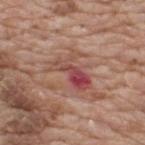Findings:
– follow-up: imaged on a skin check; not biopsied
– image-analysis metrics: a lesion area of about 9.5 mm², a shape eccentricity near 0.8, and two-axis asymmetry of about 0.45; a mean CIELAB color near L≈48 a*≈27 b*≈24 and roughly 10 lightness units darker than nearby skin; internal color variation of about 9.5 on a 0–10 scale and radial color variation of about 3.5
– anatomic site: the back
– subject: male, aged 58–62
– image source: ~15 mm tile from a whole-body skin photo
– lighting: white-light illumination